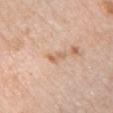Captured during whole-body skin photography for melanoma surveillance; the lesion was not biopsied.
A 15 mm close-up tile from a total-body photography series done for melanoma screening.
Captured under white-light illumination.
A female subject, aged 63 to 67.
The total-body-photography lesion software estimated a footprint of about 2.5 mm², an eccentricity of roughly 0.85, and two-axis asymmetry of about 0.45. The software also gave an average lesion color of about L≈64 a*≈19 b*≈33 (CIELAB), roughly 8 lightness units darker than nearby skin, and a normalized border contrast of about 5.5. The analysis additionally found a border-irregularity rating of about 5/10 and radial color variation of about 0.
Located on the chest.
Measured at roughly 2.5 mm in maximum diameter.